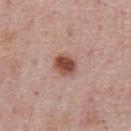Impression:
This lesion was catalogued during total-body skin photography and was not selected for biopsy.
Acquisition and patient details:
A region of skin cropped from a whole-body photographic capture, roughly 15 mm wide. An algorithmic analysis of the crop reported a footprint of about 5.5 mm² and two-axis asymmetry of about 0.15. It also reported a detector confidence of about 100 out of 100 that the crop contains a lesion. The patient is a male aged approximately 75. Measured at roughly 3 mm in maximum diameter. Imaged with white-light lighting. Located on the chest.